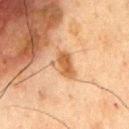Cropped from a whole-body photographic skin survey; the tile spans about 15 mm.
About 3.5 mm across.
Imaged with cross-polarized lighting.
Located on the chest.
A male patient, aged 73–77.